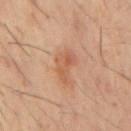Recorded during total-body skin imaging; not selected for excision or biopsy.